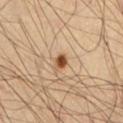lesion diameter = ≈1.5 mm
site = the chest
illumination = cross-polarized illumination
subject = male, about 55 years old
image = ~15 mm crop, total-body skin-cancer survey